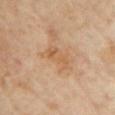Imaged during a routine full-body skin examination; the lesion was not biopsied and no histopathology is available.
The subject is a female roughly 60 years of age.
From the chest.
A close-up tile cropped from a whole-body skin photograph, about 15 mm across.